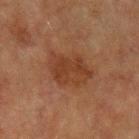Part of a total-body skin-imaging series; this lesion was reviewed on a skin check and was not flagged for biopsy.
The lesion is on the left upper arm.
A male subject, aged 73 to 77.
A lesion tile, about 15 mm wide, cut from a 3D total-body photograph.
Imaged with cross-polarized lighting.